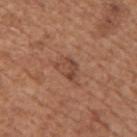Assessment:
Imaged during a routine full-body skin examination; the lesion was not biopsied and no histopathology is available.
Image and clinical context:
On the upper back. A 15 mm close-up tile from a total-body photography series done for melanoma screening. Longest diameter approximately 3.5 mm. The subject is a male approximately 65 years of age. This is a white-light tile. The total-body-photography lesion software estimated a lesion color around L≈46 a*≈22 b*≈29 in CIELAB and about 8 CIELAB-L* units darker than the surrounding skin.